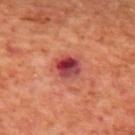Case summary:
* notes · catalogued during a skin exam; not biopsied
* patient · male, aged 68–72
* image · ~15 mm tile from a whole-body skin photo
* anatomic site · the mid back
* illumination · cross-polarized